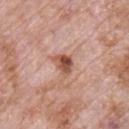Q: Is there a histopathology result?
A: catalogued during a skin exam; not biopsied
Q: What lighting was used for the tile?
A: white-light
Q: Lesion location?
A: the front of the torso
Q: Lesion size?
A: about 3 mm
Q: How was this image acquired?
A: 15 mm crop, total-body photography
Q: What did automated image analysis measure?
A: an area of roughly 5 mm² and a shape eccentricity near 0.65; a border-irregularity rating of about 3.5/10, a within-lesion color-variation index near 5.5/10, and a peripheral color-asymmetry measure near 2; a classifier nevus-likeness of about 85/100
Q: Patient demographics?
A: male, roughly 70 years of age